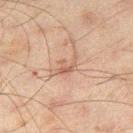No biopsy was performed on this lesion — it was imaged during a full skin examination and was not determined to be concerning. Imaged with cross-polarized lighting. The total-body-photography lesion software estimated an average lesion color of about L≈48 a*≈17 b*≈25 (CIELAB), a lesion–skin lightness drop of about 7, and a normalized lesion–skin contrast near 5.5. The recorded lesion diameter is about 3 mm. A 15 mm close-up extracted from a 3D total-body photography capture. The patient is a male approximately 45 years of age. From the right thigh.